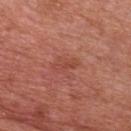The lesion was tiled from a total-body skin photograph and was not biopsied.
On the chest.
This image is a 15 mm lesion crop taken from a total-body photograph.
A male patient, in their 70s.
The total-body-photography lesion software estimated an area of roughly 3.5 mm² and an eccentricity of roughly 0.9. The analysis additionally found an average lesion color of about L≈47 a*≈28 b*≈30 (CIELAB), roughly 6 lightness units darker than nearby skin, and a normalized lesion–skin contrast near 5. It also reported a nevus-likeness score of about 0/100 and lesion-presence confidence of about 100/100.
About 3 mm across.
Imaged with white-light lighting.